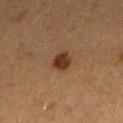Recorded during total-body skin imaging; not selected for excision or biopsy.
This is a cross-polarized tile.
The total-body-photography lesion software estimated a footprint of about 5 mm², an eccentricity of roughly 0.5, and a shape-asymmetry score of about 0.2 (0 = symmetric). And it measured an automated nevus-likeness rating near 100 out of 100 and a lesion-detection confidence of about 100/100.
The lesion's longest dimension is about 2.5 mm.
Located on the right forearm.
Cropped from a total-body skin-imaging series; the visible field is about 15 mm.
A female subject roughly 40 years of age.